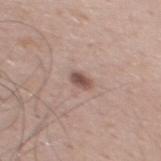The lesion was tiled from a total-body skin photograph and was not biopsied. The lesion is on the chest. Approximately 2.5 mm at its widest. A 15 mm crop from a total-body photograph taken for skin-cancer surveillance. A male subject, aged 53–57.